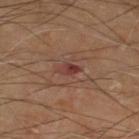Case summary:
• notes — catalogued during a skin exam; not biopsied
• image source — ~15 mm tile from a whole-body skin photo
• lesion diameter — about 3 mm
• lighting — cross-polarized
• subject — male, approximately 70 years of age
• body site — the right thigh